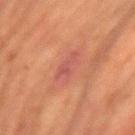{"biopsy_status": "not biopsied; imaged during a skin examination", "automated_metrics": {"border_irregularity_0_10": 3.5}, "site": "left upper arm", "lighting": "cross-polarized", "lesion_size": {"long_diameter_mm_approx": 3.5}, "patient": {"sex": "female", "age_approx": 50}, "image": {"source": "total-body photography crop", "field_of_view_mm": 15}}